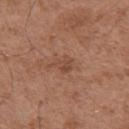Impression:
The lesion was tiled from a total-body skin photograph and was not biopsied.
Clinical summary:
A 15 mm crop from a total-body photograph taken for skin-cancer surveillance. Approximately 2.5 mm at its widest. Imaged with white-light lighting. From the left upper arm. The total-body-photography lesion software estimated an outline eccentricity of about 0.65 (0 = round, 1 = elongated) and a symmetry-axis asymmetry near 0.4. It also reported a border-irregularity index near 4/10, a within-lesion color-variation index near 2/10, and peripheral color asymmetry of about 1. The software also gave an automated nevus-likeness rating near 0 out of 100 and a detector confidence of about 100 out of 100 that the crop contains a lesion. The subject is a male aged around 50.